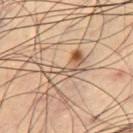Q: Is there a histopathology result?
A: total-body-photography surveillance lesion; no biopsy
Q: How was this image acquired?
A: total-body-photography crop, ~15 mm field of view
Q: What is the anatomic site?
A: the right thigh
Q: What are the patient's age and sex?
A: male, roughly 65 years of age
Q: What is the lesion's diameter?
A: ~5 mm (longest diameter)
Q: Illumination type?
A: cross-polarized